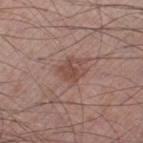Part of a total-body skin-imaging series; this lesion was reviewed on a skin check and was not flagged for biopsy. The recorded lesion diameter is about 2.5 mm. The total-body-photography lesion software estimated an area of roughly 4.5 mm² and two-axis asymmetry of about 0.4. The analysis additionally found an average lesion color of about L≈47 a*≈20 b*≈24 (CIELAB), about 9 CIELAB-L* units darker than the surrounding skin, and a normalized lesion–skin contrast near 6.5. And it measured an automated nevus-likeness rating near 15 out of 100 and a lesion-detection confidence of about 100/100. A male subject, aged around 60. The lesion is on the right thigh. The tile uses white-light illumination. A 15 mm close-up extracted from a 3D total-body photography capture.